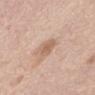Part of a total-body skin-imaging series; this lesion was reviewed on a skin check and was not flagged for biopsy.